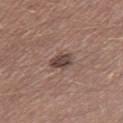- notes · no biopsy performed (imaged during a skin exam)
- lesion size · ~3 mm (longest diameter)
- TBP lesion metrics · an average lesion color of about L≈43 a*≈16 b*≈21 (CIELAB) and about 12 CIELAB-L* units darker than the surrounding skin
- image source · total-body-photography crop, ~15 mm field of view
- body site · the right thigh
- illumination · white-light
- patient · male, approximately 40 years of age